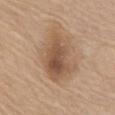notes = no biopsy performed (imaged during a skin exam)
site = the abdomen
tile lighting = white-light illumination
automated metrics = a lesion area of about 21 mm² and a symmetry-axis asymmetry near 0.25; a detector confidence of about 100 out of 100 that the crop contains a lesion
size = about 7 mm
subject = female, aged around 70
imaging modality = ~15 mm tile from a whole-body skin photo